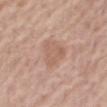The lesion was photographed on a routine skin check and not biopsied; there is no pathology result. Imaged with white-light lighting. Measured at roughly 3.5 mm in maximum diameter. The lesion-visualizer software estimated a lesion area of about 8.5 mm², an outline eccentricity of about 0.55 (0 = round, 1 = elongated), and two-axis asymmetry of about 0.35. It also reported border irregularity of about 3.5 on a 0–10 scale, a within-lesion color-variation index near 2.5/10, and radial color variation of about 0.5. And it measured a classifier nevus-likeness of about 5/100 and lesion-presence confidence of about 100/100. The patient is a male aged approximately 80. A roughly 15 mm field-of-view crop from a total-body skin photograph. Located on the leg.